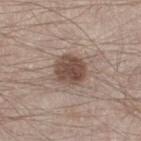Q: Was a biopsy performed?
A: imaged on a skin check; not biopsied
Q: What is the anatomic site?
A: the left thigh
Q: How was the tile lit?
A: white-light illumination
Q: What kind of image is this?
A: ~15 mm crop, total-body skin-cancer survey
Q: Patient demographics?
A: male, approximately 35 years of age
Q: How large is the lesion?
A: ~3.5 mm (longest diameter)